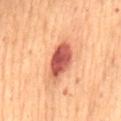  lesion_size:
    long_diameter_mm_approx: 4.5
  image:
    source: total-body photography crop
    field_of_view_mm: 15
  site: abdomen
  lighting: cross-polarized
  patient:
    sex: female
    age_approx: 80